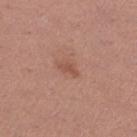Automated tile analysis of the lesion measured a lesion area of about 3 mm², a shape eccentricity near 0.8, and a symmetry-axis asymmetry near 0.35. And it measured a lesion color around L≈52 a*≈23 b*≈28 in CIELAB, a lesion–skin lightness drop of about 7, and a normalized lesion–skin contrast near 5.5.
The lesion is on the leg.
Imaged with white-light lighting.
A female subject, about 30 years old.
A 15 mm close-up extracted from a 3D total-body photography capture.